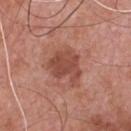{
  "biopsy_status": "not biopsied; imaged during a skin examination"
}